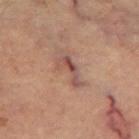Clinical impression: This lesion was catalogued during total-body skin photography and was not selected for biopsy. Image and clinical context: The tile uses cross-polarized illumination. The lesion's longest dimension is about 4 mm. On the left thigh. A roughly 15 mm field-of-view crop from a total-body skin photograph. The subject is a male in their 60s.